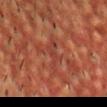Captured during whole-body skin photography for melanoma surveillance; the lesion was not biopsied. Located on the head or neck. A region of skin cropped from a whole-body photographic capture, roughly 15 mm wide. Automated image analysis of the tile measured a footprint of about 4.5 mm² and an eccentricity of roughly 0.9. The analysis additionally found roughly 5 lightness units darker than nearby skin and a normalized border contrast of about 5. It also reported a color-variation rating of about 0.5/10. Measured at roughly 3.5 mm in maximum diameter. A male patient about 55 years old. Imaged with cross-polarized lighting.